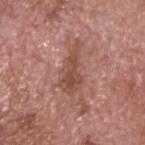patient: male, about 75 years old | site: the head or neck | size: ~6 mm (longest diameter) | lighting: white-light illumination | automated lesion analysis: an area of roughly 9.5 mm², a shape eccentricity near 0.9, and two-axis asymmetry of about 0.4; roughly 10 lightness units darker than nearby skin and a lesion-to-skin contrast of about 7.5 (normalized; higher = more distinct); a border-irregularity index near 5.5/10, a within-lesion color-variation index near 3/10, and peripheral color asymmetry of about 1 | image source: ~15 mm tile from a whole-body skin photo.